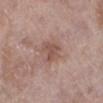| feature | finding |
|---|---|
| workup | catalogued during a skin exam; not biopsied |
| image source | total-body-photography crop, ~15 mm field of view |
| site | the right lower leg |
| illumination | white-light |
| lesion size | ~3 mm (longest diameter) |
| TBP lesion metrics | roughly 8 lightness units darker than nearby skin; border irregularity of about 3.5 on a 0–10 scale, a within-lesion color-variation index near 1.5/10, and peripheral color asymmetry of about 0.5 |
| patient | female, aged approximately 70 |